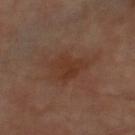{
  "biopsy_status": "not biopsied; imaged during a skin examination",
  "lighting": "cross-polarized",
  "site": "right thigh",
  "patient": {
    "sex": "male",
    "age_approx": 70
  },
  "image": {
    "source": "total-body photography crop",
    "field_of_view_mm": 15
  }
}